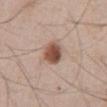Captured during whole-body skin photography for melanoma surveillance; the lesion was not biopsied.
Captured under white-light illumination.
The lesion is located on the abdomen.
The subject is a male aged around 45.
A region of skin cropped from a whole-body photographic capture, roughly 15 mm wide.
Automated image analysis of the tile measured an area of roughly 7.5 mm², an outline eccentricity of about 0.55 (0 = round, 1 = elongated), and two-axis asymmetry of about 0.15. It also reported a border-irregularity rating of about 1/10, a within-lesion color-variation index near 5.5/10, and radial color variation of about 2. And it measured a nevus-likeness score of about 100/100 and a detector confidence of about 100 out of 100 that the crop contains a lesion.